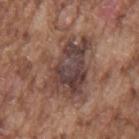Clinical impression:
No biopsy was performed on this lesion — it was imaged during a full skin examination and was not determined to be concerning.
Background:
Approximately 7.5 mm at its widest. Automated image analysis of the tile measured a mean CIELAB color near L≈42 a*≈18 b*≈21, a lesion–skin lightness drop of about 11, and a normalized lesion–skin contrast near 10. The analysis additionally found an automated nevus-likeness rating near 0 out of 100 and lesion-presence confidence of about 75/100. A male subject, approximately 75 years of age. Cropped from a total-body skin-imaging series; the visible field is about 15 mm. Located on the mid back. Captured under white-light illumination.